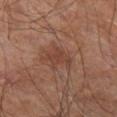Recorded during total-body skin imaging; not selected for excision or biopsy.
The tile uses cross-polarized illumination.
The lesion's longest dimension is about 3 mm.
A male patient, approximately 60 years of age.
Cropped from a total-body skin-imaging series; the visible field is about 15 mm.
From the leg.